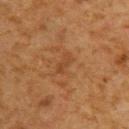follow-up=no biopsy performed (imaged during a skin exam)
image-analysis metrics=a lesion color around L≈37 a*≈20 b*≈32 in CIELAB, about 5 CIELAB-L* units darker than the surrounding skin, and a normalized border contrast of about 4.5; a border-irregularity rating of about 3/10 and peripheral color asymmetry of about 1
patient=male, roughly 60 years of age
lesion diameter=~2.5 mm (longest diameter)
location=the upper back
tile lighting=cross-polarized
image=~15 mm tile from a whole-body skin photo